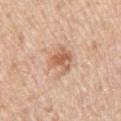<tbp_lesion>
  <biopsy_status>not biopsied; imaged during a skin examination</biopsy_status>
  <lighting>white-light</lighting>
  <patient>
    <sex>male</sex>
    <age_approx>65</age_approx>
  </patient>
  <lesion_size>
    <long_diameter_mm_approx>5.0</long_diameter_mm_approx>
  </lesion_size>
  <automated_metrics>
    <cielab_L>64</cielab_L>
    <cielab_a>20</cielab_a>
    <cielab_b>32</cielab_b>
    <vs_skin_darker_L>9.0</vs_skin_darker_L>
    <vs_skin_contrast_norm>6.0</vs_skin_contrast_norm>
    <lesion_detection_confidence_0_100>100</lesion_detection_confidence_0_100>
  </automated_metrics>
  <site>left upper arm</site>
  <image>
    <source>total-body photography crop</source>
    <field_of_view_mm>15</field_of_view_mm>
  </image>
</tbp_lesion>